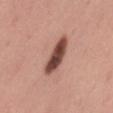biopsy status: catalogued during a skin exam; not biopsied
image: ~15 mm crop, total-body skin-cancer survey
tile lighting: white-light illumination
diameter: ≈5 mm
location: the left thigh
subject: female, aged approximately 35
automated metrics: a lesion–skin lightness drop of about 18 and a lesion-to-skin contrast of about 12 (normalized; higher = more distinct); border irregularity of about 2.5 on a 0–10 scale, a within-lesion color-variation index near 5/10, and a peripheral color-asymmetry measure near 1.5